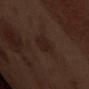| key | value |
|---|---|
| biopsy status | catalogued during a skin exam; not biopsied |
| subject | male, roughly 70 years of age |
| automated lesion analysis | an eccentricity of roughly 0.55 and a shape-asymmetry score of about 0.2 (0 = symmetric); border irregularity of about 2 on a 0–10 scale and peripheral color asymmetry of about 1 |
| tile lighting | white-light illumination |
| anatomic site | the abdomen |
| imaging modality | total-body-photography crop, ~15 mm field of view |
| size | ~3 mm (longest diameter) |